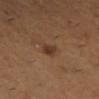Assessment: Part of a total-body skin-imaging series; this lesion was reviewed on a skin check and was not flagged for biopsy. Acquisition and patient details: From the right lower leg. The subject is a female in their mid-50s. A 15 mm crop from a total-body photograph taken for skin-cancer surveillance.